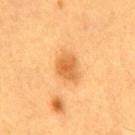The lesion was photographed on a routine skin check and not biopsied; there is no pathology result. The patient is a male in their 60s. Cropped from a whole-body photographic skin survey; the tile spans about 15 mm. Automated tile analysis of the lesion measured a lesion area of about 8 mm² and an eccentricity of roughly 0.6. The tile uses cross-polarized illumination. On the abdomen. Approximately 3.5 mm at its widest.